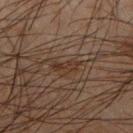<case>
  <biopsy_status>not biopsied; imaged during a skin examination</biopsy_status>
  <image>
    <source>total-body photography crop</source>
    <field_of_view_mm>15</field_of_view_mm>
  </image>
  <lesion_size>
    <long_diameter_mm_approx>3.0</long_diameter_mm_approx>
  </lesion_size>
  <patient>
    <sex>male</sex>
    <age_approx>65</age_approx>
  </patient>
  <lighting>cross-polarized</lighting>
  <site>right forearm</site>
</case>